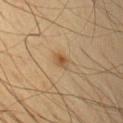Notes:
• biopsy status: total-body-photography surveillance lesion; no biopsy
• acquisition: 15 mm crop, total-body photography
• site: the arm
• patient: male, aged around 45
• size: ≈2 mm